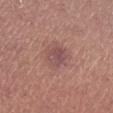Q: Was this lesion biopsied?
A: no biopsy performed (imaged during a skin exam)
Q: What did automated image analysis measure?
A: a color-variation rating of about 3.5/10
Q: What are the patient's age and sex?
A: male, approximately 65 years of age
Q: How was this image acquired?
A: ~15 mm tile from a whole-body skin photo
Q: Where on the body is the lesion?
A: the left lower leg
Q: How large is the lesion?
A: about 3.5 mm
Q: What lighting was used for the tile?
A: white-light